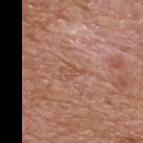This lesion was catalogued during total-body skin photography and was not selected for biopsy.
The lesion is on the upper back.
The lesion's longest dimension is about 2.5 mm.
A lesion tile, about 15 mm wide, cut from a 3D total-body photograph.
An algorithmic analysis of the crop reported a footprint of about 3 mm² and two-axis asymmetry of about 0.45. And it measured a lesion color around L≈52 a*≈23 b*≈30 in CIELAB, roughly 6 lightness units darker than nearby skin, and a normalized lesion–skin contrast near 4.5.
A male subject, aged 63 to 67.